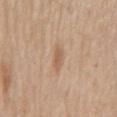<tbp_lesion>
  <lighting>white-light</lighting>
  <image>
    <source>total-body photography crop</source>
    <field_of_view_mm>15</field_of_view_mm>
  </image>
  <site>mid back</site>
  <automated_metrics>
    <area_mm2_approx>3.0</area_mm2_approx>
    <eccentricity>0.9</eccentricity>
    <shape_asymmetry>0.35</shape_asymmetry>
    <border_irregularity_0_10>3.0</border_irregularity_0_10>
  </automated_metrics>
  <patient>
    <sex>male</sex>
    <age_approx>60</age_approx>
  </patient>
  <lesion_size>
    <long_diameter_mm_approx>3.0</long_diameter_mm_approx>
  </lesion_size>
</tbp_lesion>